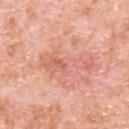biopsy status = no biopsy performed (imaged during a skin exam); imaging modality = ~15 mm crop, total-body skin-cancer survey; size = ~6.5 mm (longest diameter); subject = male, about 80 years old; location = the upper back.